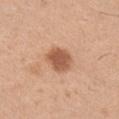Part of a total-body skin-imaging series; this lesion was reviewed on a skin check and was not flagged for biopsy. A male patient roughly 40 years of age. A region of skin cropped from a whole-body photographic capture, roughly 15 mm wide. Approximately 3.5 mm at its widest. The lesion is on the right upper arm.